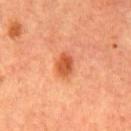notes = catalogued during a skin exam; not biopsied | location = the chest | image = ~15 mm crop, total-body skin-cancer survey | tile lighting = cross-polarized illumination | patient = male, in their mid- to late 60s.